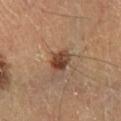Recorded during total-body skin imaging; not selected for excision or biopsy.
The lesion-visualizer software estimated roughly 12 lightness units darker than nearby skin. The analysis additionally found border irregularity of about 2 on a 0–10 scale, a within-lesion color-variation index near 5.5/10, and peripheral color asymmetry of about 2.
The tile uses cross-polarized illumination.
Cropped from a whole-body photographic skin survey; the tile spans about 15 mm.
From the right lower leg.
A male subject aged around 60.